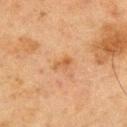<case>
<biopsy_status>not biopsied; imaged during a skin examination</biopsy_status>
<image>
  <source>total-body photography crop</source>
  <field_of_view_mm>15</field_of_view_mm>
</image>
<site>upper back</site>
<automated_metrics>
  <cielab_L>49</cielab_L>
  <cielab_a>20</cielab_a>
  <cielab_b>35</cielab_b>
  <vs_skin_contrast_norm>6.0</vs_skin_contrast_norm>
</automated_metrics>
<lesion_size>
  <long_diameter_mm_approx>2.5</long_diameter_mm_approx>
</lesion_size>
<lighting>cross-polarized</lighting>
<patient>
  <sex>male</sex>
  <age_approx>70</age_approx>
</patient>
</case>